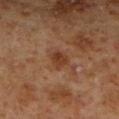workup: total-body-photography surveillance lesion; no biopsy | image-analysis metrics: a footprint of about 3.5 mm², a shape eccentricity near 0.6, and a shape-asymmetry score of about 0.25 (0 = symmetric); a lesion color around L≈35 a*≈21 b*≈30 in CIELAB and a normalized lesion–skin contrast near 7.5; border irregularity of about 2 on a 0–10 scale; a classifier nevus-likeness of about 25/100 and a detector confidence of about 100 out of 100 that the crop contains a lesion | illumination: cross-polarized illumination | anatomic site: the right lower leg | imaging modality: ~15 mm crop, total-body skin-cancer survey | lesion diameter: ≈2.5 mm | patient: male, aged approximately 60.